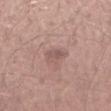biopsy_status: not biopsied; imaged during a skin examination
patient:
  sex: male
  age_approx: 45
lighting: white-light
site: left forearm
lesion_size:
  long_diameter_mm_approx: 2.5
image:
  source: total-body photography crop
  field_of_view_mm: 15